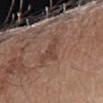Recorded during total-body skin imaging; not selected for excision or biopsy. Captured under white-light illumination. A 15 mm crop from a total-body photograph taken for skin-cancer surveillance. The patient is a male roughly 70 years of age. The lesion-visualizer software estimated a lesion area of about 4 mm² and a shape-asymmetry score of about 0.6 (0 = symmetric). It also reported a lesion color around L≈43 a*≈19 b*≈25 in CIELAB, a lesion–skin lightness drop of about 7, and a normalized border contrast of about 6. It also reported a border-irregularity index near 7/10, internal color variation of about 1 on a 0–10 scale, and peripheral color asymmetry of about 0.5. The analysis additionally found a nevus-likeness score of about 0/100. Located on the arm.